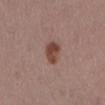| field | value |
|---|---|
| follow-up | imaged on a skin check; not biopsied |
| subject | male, roughly 55 years of age |
| lesion size | ~3.5 mm (longest diameter) |
| acquisition | ~15 mm tile from a whole-body skin photo |
| lighting | white-light |
| location | the mid back |
| TBP lesion metrics | a lesion area of about 6 mm²; a classifier nevus-likeness of about 95/100 |